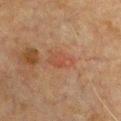Case summary:
– lighting: cross-polarized
– patient: male, aged 63 to 67
– image-analysis metrics: an area of roughly 5 mm², a shape eccentricity near 0.85, and a symmetry-axis asymmetry near 0.25; a mean CIELAB color near L≈39 a*≈21 b*≈27, roughly 5 lightness units darker than nearby skin, and a normalized border contrast of about 5; an automated nevus-likeness rating near 15 out of 100 and a detector confidence of about 100 out of 100 that the crop contains a lesion
– anatomic site: the front of the torso
– lesion size: ≈3.5 mm
– image source: ~15 mm tile from a whole-body skin photo